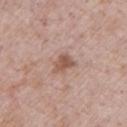{"biopsy_status": "not biopsied; imaged during a skin examination", "patient": {"sex": "male", "age_approx": 55}, "automated_metrics": {"eccentricity": 0.65, "shape_asymmetry": 0.3, "vs_skin_darker_L": 11.0}, "image": {"source": "total-body photography crop", "field_of_view_mm": 15}, "lesion_size": {"long_diameter_mm_approx": 3.0}}